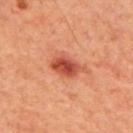Assessment: Imaged during a routine full-body skin examination; the lesion was not biopsied and no histopathology is available. Context: Automated image analysis of the tile measured an area of roughly 7 mm² and an outline eccentricity of about 0.75 (0 = round, 1 = elongated). It also reported a border-irregularity rating of about 2.5/10, internal color variation of about 5 on a 0–10 scale, and radial color variation of about 1.5. A roughly 15 mm field-of-view crop from a total-body skin photograph. A male subject, aged around 70. From the upper back. Imaged with cross-polarized lighting. Longest diameter approximately 3.5 mm.